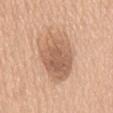Imaged during a routine full-body skin examination; the lesion was not biopsied and no histopathology is available. The subject is a female aged approximately 60. This image is a 15 mm lesion crop taken from a total-body photograph. Captured under white-light illumination. From the mid back.